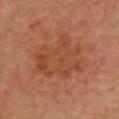notes — total-body-photography surveillance lesion; no biopsy | patient — female, approximately 40 years of age | body site — the chest | image — 15 mm crop, total-body photography | diameter — ≈7 mm.